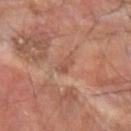Findings:
• biopsy status · catalogued during a skin exam; not biopsied
• imaging modality · total-body-photography crop, ~15 mm field of view
• diameter · about 3 mm
• subject · male, roughly 65 years of age
• location · the left forearm